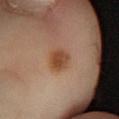Assessment:
Imaged during a routine full-body skin examination; the lesion was not biopsied and no histopathology is available.
Clinical summary:
A 15 mm close-up tile from a total-body photography series done for melanoma screening. Captured under cross-polarized illumination. The lesion-visualizer software estimated a lesion area of about 7 mm², a shape eccentricity near 0.55, and two-axis asymmetry of about 0.15. From the right lower leg. The subject is a female aged around 45. About 3 mm across.